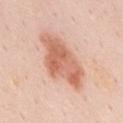follow-up = catalogued during a skin exam; not biopsied | subject = male, aged approximately 50 | image source = ~15 mm crop, total-body skin-cancer survey | anatomic site = the mid back.